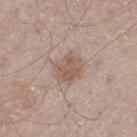workup=catalogued during a skin exam; not biopsied
location=the left thigh
patient=male, in their mid- to late 60s
lighting=white-light illumination
imaging modality=~15 mm crop, total-body skin-cancer survey
size=≈3.5 mm